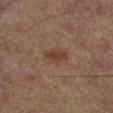Impression: Part of a total-body skin-imaging series; this lesion was reviewed on a skin check and was not flagged for biopsy. Context: The lesion's longest dimension is about 3 mm. The lesion is located on the left lower leg. This is a cross-polarized tile. Automated image analysis of the tile measured an area of roughly 5 mm², an outline eccentricity of about 0.8 (0 = round, 1 = elongated), and a shape-asymmetry score of about 0.2 (0 = symmetric). The subject is a male aged approximately 65. A 15 mm close-up tile from a total-body photography series done for melanoma screening.